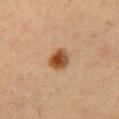Findings:
- follow-up: catalogued during a skin exam; not biopsied
- imaging modality: ~15 mm tile from a whole-body skin photo
- patient: female, approximately 40 years of age
- location: the chest